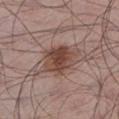{"biopsy_status": "not biopsied; imaged during a skin examination", "automated_metrics": {"eccentricity": 0.65, "cielab_L": 45, "cielab_a": 19, "cielab_b": 24, "vs_skin_darker_L": 12.0, "vs_skin_contrast_norm": 9.0, "lesion_detection_confidence_0_100": 100}, "image": {"source": "total-body photography crop", "field_of_view_mm": 15}, "patient": {"sex": "male", "age_approx": 55}, "site": "leg", "lighting": "white-light"}